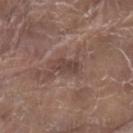notes=imaged on a skin check; not biopsied | lesion diameter=about 3 mm | lighting=white-light | location=the left lower leg | patient=male, aged approximately 80 | imaging modality=~15 mm tile from a whole-body skin photo.